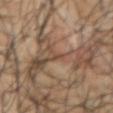<tbp_lesion>
<biopsy_status>not biopsied; imaged during a skin examination</biopsy_status>
<lesion_size>
  <long_diameter_mm_approx>1.5</long_diameter_mm_approx>
</lesion_size>
<patient>
  <sex>male</sex>
  <age_approx>40</age_approx>
</patient>
<site>arm</site>
<lighting>cross-polarized</lighting>
<image>
  <source>total-body photography crop</source>
  <field_of_view_mm>15</field_of_view_mm>
</image>
<automated_metrics>
  <cielab_L>46</cielab_L>
  <cielab_a>18</cielab_a>
  <cielab_b>29</cielab_b>
  <vs_skin_darker_L>7.0</vs_skin_darker_L>
</automated_metrics>
</tbp_lesion>